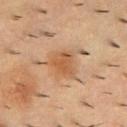Clinical impression: Imaged during a routine full-body skin examination; the lesion was not biopsied and no histopathology is available. Acquisition and patient details: Captured under cross-polarized illumination. The recorded lesion diameter is about 4 mm. The subject is a male aged approximately 55. From the back. A close-up tile cropped from a whole-body skin photograph, about 15 mm across.